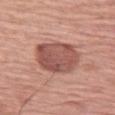Q: Was a biopsy performed?
A: no biopsy performed (imaged during a skin exam)
Q: What is the anatomic site?
A: the left thigh
Q: How was this image acquired?
A: ~15 mm crop, total-body skin-cancer survey
Q: What did automated image analysis measure?
A: a shape eccentricity near 0.6 and a symmetry-axis asymmetry near 0.1; an average lesion color of about L≈52 a*≈25 b*≈25 (CIELAB) and roughly 13 lightness units darker than nearby skin; an automated nevus-likeness rating near 85 out of 100 and a lesion-detection confidence of about 100/100
Q: How was the tile lit?
A: white-light
Q: What is the lesion's diameter?
A: about 5.5 mm
Q: Patient demographics?
A: female, approximately 65 years of age